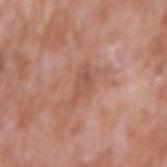follow-up — total-body-photography surveillance lesion; no biopsy
image source — ~15 mm tile from a whole-body skin photo
patient — male, aged 58 to 62
anatomic site — the arm